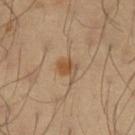Case summary:
* subject · male, aged approximately 40
* illumination · cross-polarized
* imaging modality · 15 mm crop, total-body photography
* lesion size · ~2.5 mm (longest diameter)
* anatomic site · the arm